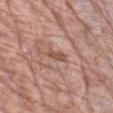No biopsy was performed on this lesion — it was imaged during a full skin examination and was not determined to be concerning. A male subject in their mid- to late 70s. A roughly 15 mm field-of-view crop from a total-body skin photograph. Imaged with white-light lighting. From the chest. An algorithmic analysis of the crop reported a footprint of about 3 mm², an eccentricity of roughly 0.9, and two-axis asymmetry of about 0.2. The software also gave an average lesion color of about L≈52 a*≈22 b*≈27 (CIELAB), roughly 9 lightness units darker than nearby skin, and a lesion-to-skin contrast of about 7 (normalized; higher = more distinct). It also reported an automated nevus-likeness rating near 0 out of 100 and a detector confidence of about 65 out of 100 that the crop contains a lesion. The lesion's longest dimension is about 3 mm.